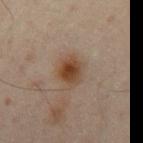Recorded during total-body skin imaging; not selected for excision or biopsy. The lesion's longest dimension is about 4 mm. The total-body-photography lesion software estimated a lesion color around L≈39 a*≈16 b*≈27 in CIELAB and a normalized lesion–skin contrast near 9.5. The analysis additionally found a border-irregularity index near 2/10, a within-lesion color-variation index near 5.5/10, and radial color variation of about 1.5. The analysis additionally found an automated nevus-likeness rating near 95 out of 100 and a detector confidence of about 100 out of 100 that the crop contains a lesion. The lesion is located on the left upper arm. A male subject aged 48 to 52. A 15 mm close-up tile from a total-body photography series done for melanoma screening.